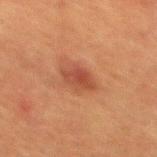workup: catalogued during a skin exam; not biopsied | acquisition: ~15 mm crop, total-body skin-cancer survey | illumination: cross-polarized | automated lesion analysis: a lesion area of about 6.5 mm² and an eccentricity of roughly 0.6; border irregularity of about 2.5 on a 0–10 scale, a within-lesion color-variation index near 3/10, and radial color variation of about 1 | subject: male, aged 48 to 52 | body site: the mid back.